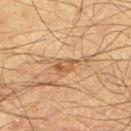Q: Was this lesion biopsied?
A: total-body-photography surveillance lesion; no biopsy
Q: Lesion location?
A: the left thigh
Q: What are the patient's age and sex?
A: male, aged approximately 65
Q: What is the imaging modality?
A: 15 mm crop, total-body photography
Q: How was the tile lit?
A: cross-polarized
Q: What is the lesion's diameter?
A: about 2.5 mm